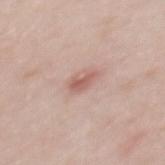- follow-up · total-body-photography surveillance lesion; no biopsy
- tile lighting · white-light
- lesion size · about 3 mm
- patient · female, aged approximately 30
- acquisition · 15 mm crop, total-body photography
- site · the mid back